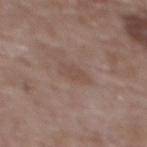Clinical impression: The lesion was tiled from a total-body skin photograph and was not biopsied.